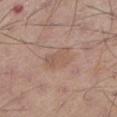  biopsy_status: not biopsied; imaged during a skin examination
  automated_metrics:
    area_mm2_approx: 7.0
    eccentricity: 0.75
    shape_asymmetry: 0.15
    cielab_L: 55
    cielab_a: 18
    cielab_b: 26
    vs_skin_darker_L: 6.0
    vs_skin_contrast_norm: 4.5
    border_irregularity_0_10: 2.0
    color_variation_0_10: 2.5
    peripheral_color_asymmetry: 1.0
    nevus_likeness_0_100: 0
    lesion_detection_confidence_0_100: 100
  lesion_size:
    long_diameter_mm_approx: 3.5
  site: left lower leg
  patient:
    sex: male
    age_approx: 55
  image:
    source: total-body photography crop
    field_of_view_mm: 15